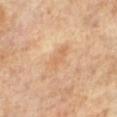Q: Is there a histopathology result?
A: catalogued during a skin exam; not biopsied
Q: Patient demographics?
A: female, roughly 65 years of age
Q: How large is the lesion?
A: ≈3.5 mm
Q: Lesion location?
A: the right leg
Q: What kind of image is this?
A: ~15 mm tile from a whole-body skin photo
Q: How was the tile lit?
A: cross-polarized